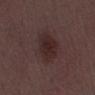Background:
A close-up tile cropped from a whole-body skin photograph, about 15 mm across. The lesion-visualizer software estimated a lesion area of about 11 mm², a shape eccentricity near 0.9, and two-axis asymmetry of about 0.2. It also reported an average lesion color of about L≈25 a*≈16 b*≈16 (CIELAB) and roughly 7 lightness units darker than nearby skin. It also reported border irregularity of about 2.5 on a 0–10 scale, internal color variation of about 2.5 on a 0–10 scale, and radial color variation of about 1. Captured under white-light illumination. The patient is a male aged around 50. The recorded lesion diameter is about 5.5 mm.